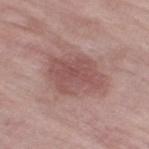This lesion was catalogued during total-body skin photography and was not selected for biopsy. The patient is a female aged 68 to 72. The lesion's longest dimension is about 5.5 mm. An algorithmic analysis of the crop reported an area of roughly 17 mm², an eccentricity of roughly 0.75, and a symmetry-axis asymmetry near 0.3. The software also gave a lesion color around L≈51 a*≈22 b*≈21 in CIELAB, about 9 CIELAB-L* units darker than the surrounding skin, and a lesion-to-skin contrast of about 6.5 (normalized; higher = more distinct). The lesion is on the right thigh. The tile uses white-light illumination. A close-up tile cropped from a whole-body skin photograph, about 15 mm across.